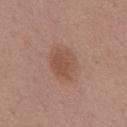The lesion was tiled from a total-body skin photograph and was not biopsied.
Captured under white-light illumination.
On the back.
The subject is a female aged 48–52.
The lesion-visualizer software estimated a mean CIELAB color near L≈51 a*≈20 b*≈28, roughly 8 lightness units darker than nearby skin, and a lesion-to-skin contrast of about 6.5 (normalized; higher = more distinct).
A 15 mm crop from a total-body photograph taken for skin-cancer surveillance.